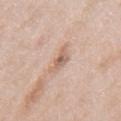No biopsy was performed on this lesion — it was imaged during a full skin examination and was not determined to be concerning. The lesion is on the mid back. About 3.5 mm across. The patient is a male aged around 65. Cropped from a whole-body photographic skin survey; the tile spans about 15 mm.